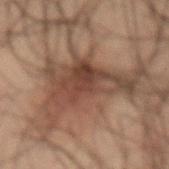biopsy status — total-body-photography surveillance lesion; no biopsy | subject — male, aged around 50 | image source — total-body-photography crop, ~15 mm field of view | diameter — about 8 mm | location — the lower back.